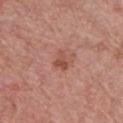The lesion was photographed on a routine skin check and not biopsied; there is no pathology result.
Captured under white-light illumination.
A male patient, about 50 years old.
A 15 mm close-up extracted from a 3D total-body photography capture.
The lesion is located on the chest.
The lesion's longest dimension is about 2.5 mm.